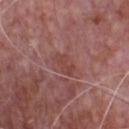<tbp_lesion>
<biopsy_status>not biopsied; imaged during a skin examination</biopsy_status>
<lighting>white-light</lighting>
<site>front of the torso</site>
<lesion_size>
  <long_diameter_mm_approx>3.0</long_diameter_mm_approx>
</lesion_size>
<image>
  <source>total-body photography crop</source>
  <field_of_view_mm>15</field_of_view_mm>
</image>
<patient>
  <sex>male</sex>
  <age_approx>70</age_approx>
</patient>
</tbp_lesion>